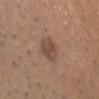follow-up=no biopsy performed (imaged during a skin exam)
illumination=white-light illumination
acquisition=15 mm crop, total-body photography
body site=the head or neck
patient=male, in their mid-20s
image-analysis metrics=an eccentricity of roughly 0.8 and a symmetry-axis asymmetry near 0.2; border irregularity of about 2 on a 0–10 scale, internal color variation of about 3 on a 0–10 scale, and peripheral color asymmetry of about 1